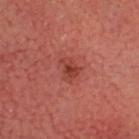{"biopsy_status": "not biopsied; imaged during a skin examination"}